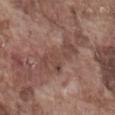Clinical impression: Recorded during total-body skin imaging; not selected for excision or biopsy. Background: A 15 mm close-up extracted from a 3D total-body photography capture. Measured at roughly 5.5 mm in maximum diameter. The patient is a male aged approximately 75. Located on the abdomen.